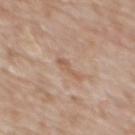Background: A female subject about 85 years old or older. A roughly 15 mm field-of-view crop from a total-body skin photograph. On the upper back. Captured under white-light illumination. Measured at roughly 3 mm in maximum diameter.